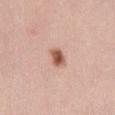This lesion was catalogued during total-body skin photography and was not selected for biopsy. Imaged with white-light lighting. On the abdomen. Approximately 2.5 mm at its widest. A region of skin cropped from a whole-body photographic capture, roughly 15 mm wide. A female patient aged 23 to 27.